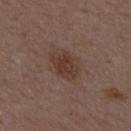No biopsy was performed on this lesion — it was imaged during a full skin examination and was not determined to be concerning. A male subject in their 50s. The tile uses white-light illumination. Measured at roughly 4 mm in maximum diameter. Located on the mid back. The total-body-photography lesion software estimated a footprint of about 8.5 mm², a shape eccentricity near 0.65, and two-axis asymmetry of about 0.15. The analysis additionally found a border-irregularity index near 2/10, a color-variation rating of about 2.5/10, and radial color variation of about 1. A close-up tile cropped from a whole-body skin photograph, about 15 mm across.